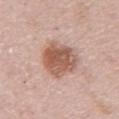image source = ~15 mm crop, total-body skin-cancer survey | anatomic site = the left upper arm | patient = female, aged around 50.